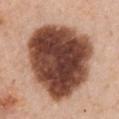Imaged during a routine full-body skin examination; the lesion was not biopsied and no histopathology is available. Imaged with white-light lighting. The lesion is located on the chest. A female subject aged around 60. A lesion tile, about 15 mm wide, cut from a 3D total-body photograph.